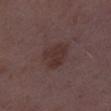The lesion was tiled from a total-body skin photograph and was not biopsied. This is a white-light tile. A female subject, in their mid- to late 30s. The lesion is located on the right thigh. The recorded lesion diameter is about 4 mm. Automated tile analysis of the lesion measured a border-irregularity index near 2/10. Cropped from a total-body skin-imaging series; the visible field is about 15 mm.